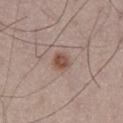acquisition=total-body-photography crop, ~15 mm field of view | body site=the right thigh | patient=male, aged around 75.